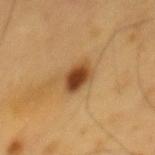Q: Was this lesion biopsied?
A: no biopsy performed (imaged during a skin exam)
Q: What is the lesion's diameter?
A: ~3.5 mm (longest diameter)
Q: How was the tile lit?
A: cross-polarized
Q: Lesion location?
A: the mid back
Q: How was this image acquired?
A: ~15 mm tile from a whole-body skin photo
Q: Patient demographics?
A: male, aged 58–62
Q: What did automated image analysis measure?
A: a lesion color around L≈45 a*≈22 b*≈38 in CIELAB and a lesion–skin lightness drop of about 16; border irregularity of about 2 on a 0–10 scale, internal color variation of about 5 on a 0–10 scale, and radial color variation of about 2